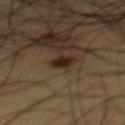No biopsy was performed on this lesion — it was imaged during a full skin examination and was not determined to be concerning.
The lesion-visualizer software estimated an eccentricity of roughly 0.4 and two-axis asymmetry of about 0.25. And it measured a lesion color around L≈18 a*≈12 b*≈18 in CIELAB, roughly 8 lightness units darker than nearby skin, and a normalized border contrast of about 11. The analysis additionally found a border-irregularity index near 2/10, a within-lesion color-variation index near 2/10, and peripheral color asymmetry of about 0.5. The software also gave a classifier nevus-likeness of about 95/100 and lesion-presence confidence of about 100/100.
The lesion is on the mid back.
This image is a 15 mm lesion crop taken from a total-body photograph.
The recorded lesion diameter is about 2.5 mm.
Captured under cross-polarized illumination.
A male subject aged 58 to 62.